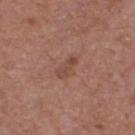This lesion was catalogued during total-body skin photography and was not selected for biopsy. On the leg. Captured under white-light illumination. A roughly 15 mm field-of-view crop from a total-body skin photograph. The patient is a female in their 40s.